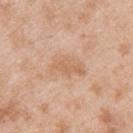<lesion>
<biopsy_status>not biopsied; imaged during a skin examination</biopsy_status>
<site>back</site>
<patient>
  <sex>male</sex>
  <age_approx>25</age_approx>
</patient>
<lighting>white-light</lighting>
<lesion_size>
  <long_diameter_mm_approx>4.5</long_diameter_mm_approx>
</lesion_size>
<image>
  <source>total-body photography crop</source>
  <field_of_view_mm>15</field_of_view_mm>
</image>
</lesion>